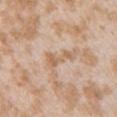Notes:
• notes: imaged on a skin check; not biopsied
• lesion diameter: ~3.5 mm (longest diameter)
• illumination: white-light illumination
• body site: the left upper arm
• image: 15 mm crop, total-body photography
• subject: female, in their mid- to late 20s
• TBP lesion metrics: a footprint of about 4.5 mm², an eccentricity of roughly 0.9, and two-axis asymmetry of about 0.6; a mean CIELAB color near L≈62 a*≈17 b*≈32, roughly 8 lightness units darker than nearby skin, and a normalized border contrast of about 6; a nevus-likeness score of about 0/100 and a lesion-detection confidence of about 95/100